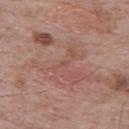notes — imaged on a skin check; not biopsied | anatomic site — the upper back | lesion diameter — about 1 mm | illumination — white-light illumination | patient — male, aged approximately 70 | TBP lesion metrics — a footprint of about 0.5 mm² and a shape eccentricity near 0.75; roughly 4 lightness units darker than nearby skin; a border-irregularity rating of about 3.5/10 and a within-lesion color-variation index near 0/10; a lesion-detection confidence of about 95/100 | image — ~15 mm crop, total-body skin-cancer survey.